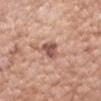notes — total-body-photography surveillance lesion; no biopsy
patient — female, roughly 70 years of age
lesion size — about 3.5 mm
image — ~15 mm crop, total-body skin-cancer survey
site — the arm
lighting — white-light illumination